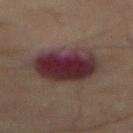follow-up: total-body-photography surveillance lesion; no biopsy
automated metrics: a lesion area of about 25 mm² and two-axis asymmetry of about 0.15; a border-irregularity index near 2/10, a color-variation rating of about 5.5/10, and peripheral color asymmetry of about 1.5; a classifier nevus-likeness of about 0/100 and lesion-presence confidence of about 100/100
body site: the abdomen
image: total-body-photography crop, ~15 mm field of view
lesion size: about 6.5 mm
illumination: cross-polarized illumination
subject: male, in their 70s